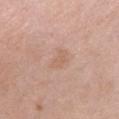| field | value |
|---|---|
| follow-up | catalogued during a skin exam; not biopsied |
| diameter | about 2.5 mm |
| imaging modality | total-body-photography crop, ~15 mm field of view |
| patient | female, aged around 35 |
| location | the front of the torso |